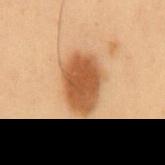Impression:
Recorded during total-body skin imaging; not selected for excision or biopsy.
Clinical summary:
Cropped from a whole-body photographic skin survey; the tile spans about 15 mm. A male subject, approximately 55 years of age. Captured under cross-polarized illumination. Automated tile analysis of the lesion measured an eccentricity of roughly 0.8 and a symmetry-axis asymmetry near 0.15. The software also gave a lesion color around L≈45 a*≈20 b*≈33 in CIELAB, roughly 13 lightness units darker than nearby skin, and a normalized lesion–skin contrast near 10. And it measured border irregularity of about 2 on a 0–10 scale and a color-variation rating of about 2.5/10. The analysis additionally found an automated nevus-likeness rating near 100 out of 100 and lesion-presence confidence of about 100/100. The lesion is located on the mid back. The lesion's longest dimension is about 6 mm.